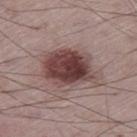The lesion was tiled from a total-body skin photograph and was not biopsied. The lesion is located on the right thigh. Captured under white-light illumination. A male patient, about 75 years old. Cropped from a whole-body photographic skin survey; the tile spans about 15 mm.